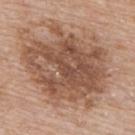{"biopsy_status": "not biopsied; imaged during a skin examination", "lesion_size": {"long_diameter_mm_approx": 10.5}, "site": "upper back", "image": {"source": "total-body photography crop", "field_of_view_mm": 15}, "automated_metrics": {"area_mm2_approx": 65.0, "eccentricity": 0.55, "shape_asymmetry": 0.25, "cielab_L": 52, "cielab_a": 19, "cielab_b": 29, "vs_skin_darker_L": 12.0, "vs_skin_contrast_norm": 8.0, "nevus_likeness_0_100": 20}, "patient": {"sex": "male", "age_approx": 65}}